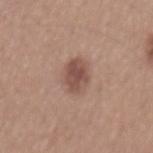{
  "image": {
    "source": "total-body photography crop",
    "field_of_view_mm": 15
  },
  "site": "mid back",
  "patient": {
    "sex": "male",
    "age_approx": 55
  }
}